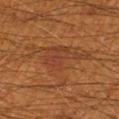A male patient approximately 60 years of age.
Cropped from a total-body skin-imaging series; the visible field is about 15 mm.
From the right lower leg.
About 8 mm across.